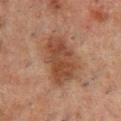Impression: This lesion was catalogued during total-body skin photography and was not selected for biopsy. Background: A lesion tile, about 15 mm wide, cut from a 3D total-body photograph. The subject is a male aged around 50. From the front of the torso. The lesion's longest dimension is about 6.5 mm.